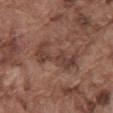The lesion was tiled from a total-body skin photograph and was not biopsied.
A male patient about 75 years old.
This is a white-light tile.
Automated image analysis of the tile measured a lesion area of about 12 mm². The software also gave an average lesion color of about L≈41 a*≈19 b*≈24 (CIELAB) and a lesion–skin lightness drop of about 8.
A 15 mm close-up extracted from a 3D total-body photography capture.
Approximately 6 mm at its widest.
The lesion is located on the mid back.